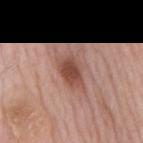<lesion>
<biopsy_status>not biopsied; imaged during a skin examination</biopsy_status>
<image>
  <source>total-body photography crop</source>
  <field_of_view_mm>15</field_of_view_mm>
</image>
<site>mid back</site>
<lesion_size>
  <long_diameter_mm_approx>4.5</long_diameter_mm_approx>
</lesion_size>
<patient>
  <sex>male</sex>
  <age_approx>60</age_approx>
</patient>
<lighting>white-light</lighting>
<automated_metrics>
  <eccentricity>0.8</eccentricity>
  <shape_asymmetry>0.25</shape_asymmetry>
  <vs_skin_darker_L>12.0</vs_skin_darker_L>
  <vs_skin_contrast_norm>8.5</vs_skin_contrast_norm>
  <border_irregularity_0_10>2.5</border_irregularity_0_10>
  <color_variation_0_10>4.0</color_variation_0_10>
</automated_metrics>
</lesion>